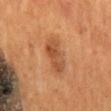Assessment: The lesion was tiled from a total-body skin photograph and was not biopsied. Image and clinical context: The subject is a male about 55 years old. Automated image analysis of the tile measured a footprint of about 9 mm², a shape eccentricity near 0.9, and a shape-asymmetry score of about 0.2 (0 = symmetric). And it measured an average lesion color of about L≈47 a*≈23 b*≈36 (CIELAB), about 9 CIELAB-L* units darker than the surrounding skin, and a normalized border contrast of about 7. It also reported a border-irregularity rating of about 2.5/10, internal color variation of about 4 on a 0–10 scale, and a peripheral color-asymmetry measure near 1.5. The software also gave an automated nevus-likeness rating near 40 out of 100 and a lesion-detection confidence of about 100/100. On the mid back. Imaged with cross-polarized lighting. Measured at roughly 5 mm in maximum diameter. A close-up tile cropped from a whole-body skin photograph, about 15 mm across.